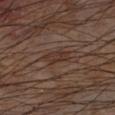Impression: No biopsy was performed on this lesion — it was imaged during a full skin examination and was not determined to be concerning. Context: A male subject roughly 65 years of age. Automated image analysis of the tile measured a mean CIELAB color near L≈32 a*≈17 b*≈22, a lesion–skin lightness drop of about 7, and a lesion-to-skin contrast of about 6.5 (normalized; higher = more distinct). The software also gave a nevus-likeness score of about 0/100 and a detector confidence of about 95 out of 100 that the crop contains a lesion. On the left forearm. Measured at roughly 3 mm in maximum diameter. A region of skin cropped from a whole-body photographic capture, roughly 15 mm wide.